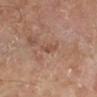Captured during whole-body skin photography for melanoma surveillance; the lesion was not biopsied. The patient is a male aged 68–72. The lesion's longest dimension is about 3 mm. The lesion is on the leg. A close-up tile cropped from a whole-body skin photograph, about 15 mm across. Captured under cross-polarized illumination.